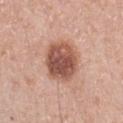Assessment: The lesion was tiled from a total-body skin photograph and was not biopsied. Background: The lesion is on the left forearm. A female subject aged around 40. The total-body-photography lesion software estimated a lesion color around L≈54 a*≈23 b*≈28 in CIELAB and a normalized border contrast of about 10. It also reported border irregularity of about 1.5 on a 0–10 scale, a color-variation rating of about 5.5/10, and peripheral color asymmetry of about 1.5. And it measured a nevus-likeness score of about 75/100. Longest diameter approximately 5 mm. Imaged with white-light lighting. A lesion tile, about 15 mm wide, cut from a 3D total-body photograph.